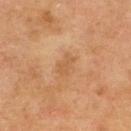Impression: This lesion was catalogued during total-body skin photography and was not selected for biopsy. Context: A female patient, aged 53 to 57. Automated image analysis of the tile measured roughly 6 lightness units darker than nearby skin and a lesion-to-skin contrast of about 4.5 (normalized; higher = more distinct). The analysis additionally found a classifier nevus-likeness of about 0/100 and lesion-presence confidence of about 100/100. A 15 mm close-up tile from a total-body photography series done for melanoma screening. Approximately 2.5 mm at its widest. Located on the upper back. The tile uses cross-polarized illumination.